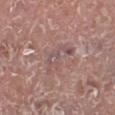{
  "biopsy_status": "not biopsied; imaged during a skin examination",
  "site": "right lower leg",
  "patient": {
    "sex": "male",
    "age_approx": 75
  },
  "image": {
    "source": "total-body photography crop",
    "field_of_view_mm": 15
  }
}